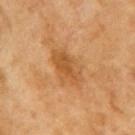| feature | finding |
|---|---|
| biopsy status | total-body-photography surveillance lesion; no biopsy |
| illumination | cross-polarized |
| patient | female, aged 68 to 72 |
| lesion diameter | ~5 mm (longest diameter) |
| imaging modality | ~15 mm tile from a whole-body skin photo |
| body site | the right upper arm |
| image-analysis metrics | a border-irregularity index near 2.5/10, a color-variation rating of about 4/10, and radial color variation of about 1.5 |